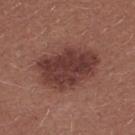Clinical impression:
Part of a total-body skin-imaging series; this lesion was reviewed on a skin check and was not flagged for biopsy.
Context:
Automated image analysis of the tile measured a lesion color around L≈38 a*≈23 b*≈22 in CIELAB, a lesion–skin lightness drop of about 11, and a normalized border contrast of about 9.5. The analysis additionally found a nevus-likeness score of about 65/100 and a lesion-detection confidence of about 100/100. The lesion's longest dimension is about 7.5 mm. Captured under white-light illumination. The lesion is on the back. A female subject, in their mid- to late 20s. A lesion tile, about 15 mm wide, cut from a 3D total-body photograph.